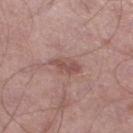Background:
Located on the left lower leg. Captured under white-light illumination. An algorithmic analysis of the crop reported roughly 9 lightness units darker than nearby skin and a lesion-to-skin contrast of about 6.5 (normalized; higher = more distinct). And it measured a color-variation rating of about 2.5/10 and radial color variation of about 1. A 15 mm close-up tile from a total-body photography series done for melanoma screening. A male subject aged around 55.